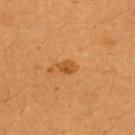This lesion was catalogued during total-body skin photography and was not selected for biopsy. About 2.5 mm across. The subject is a female in their 40s. Cropped from a total-body skin-imaging series; the visible field is about 15 mm. Located on the upper back. The tile uses cross-polarized illumination.